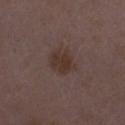- image — ~15 mm crop, total-body skin-cancer survey
- image-analysis metrics — an average lesion color of about L≈32 a*≈15 b*≈20 (CIELAB), a lesion–skin lightness drop of about 7, and a lesion-to-skin contrast of about 7.5 (normalized; higher = more distinct)
- patient — female, aged around 30
- size — about 3 mm
- anatomic site — the arm